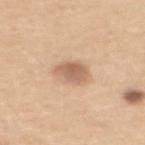Assessment: The lesion was tiled from a total-body skin photograph and was not biopsied. Acquisition and patient details: About 3.5 mm across. The subject is a female aged approximately 45. A 15 mm close-up tile from a total-body photography series done for melanoma screening. This is a white-light tile. An algorithmic analysis of the crop reported a lesion color around L≈62 a*≈19 b*≈32 in CIELAB and a lesion–skin lightness drop of about 12. The analysis additionally found a border-irregularity index near 2.5/10, internal color variation of about 3 on a 0–10 scale, and radial color variation of about 1. The software also gave lesion-presence confidence of about 100/100. Located on the back.